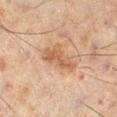Captured during whole-body skin photography for melanoma surveillance; the lesion was not biopsied. A 15 mm close-up tile from a total-body photography series done for melanoma screening. Located on the right lower leg. A male subject aged 43 to 47. Approximately 5 mm at its widest. Captured under cross-polarized illumination.